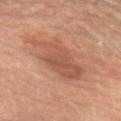  biopsy_status: not biopsied; imaged during a skin examination
  site: arm
  lighting: cross-polarized
  image:
    source: total-body photography crop
    field_of_view_mm: 15
  lesion_size:
    long_diameter_mm_approx: 6.0
  patient:
    sex: female
    age_approx: 65